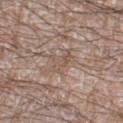Findings:
• notes · total-body-photography surveillance lesion; no biopsy
• illumination · white-light
• location · the right lower leg
• image source · ~15 mm tile from a whole-body skin photo
• automated lesion analysis · an area of roughly 4 mm²; a mean CIELAB color near L≈53 a*≈16 b*≈25 and a normalized border contrast of about 5; a color-variation rating of about 0/10 and peripheral color asymmetry of about 0
• lesion size · ≈3.5 mm
• patient · male, about 60 years old